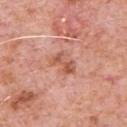| key | value |
|---|---|
| body site | the chest |
| image | ~15 mm tile from a whole-body skin photo |
| TBP lesion metrics | a lesion area of about 4.5 mm² and a shape eccentricity near 0.85 |
| lesion size | about 3 mm |
| lighting | white-light |
| patient | male, in their 80s |